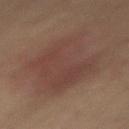<case>
<image>
  <source>total-body photography crop</source>
  <field_of_view_mm>15</field_of_view_mm>
</image>
<site>lower back</site>
<patient>
  <sex>male</sex>
  <age_approx>65</age_approx>
</patient>
</case>